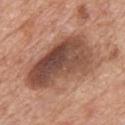Clinical impression:
Part of a total-body skin-imaging series; this lesion was reviewed on a skin check and was not flagged for biopsy.
Clinical summary:
A male patient aged 58–62. The total-body-photography lesion software estimated an eccentricity of roughly 0.85 and a shape-asymmetry score of about 0.3 (0 = symmetric). It also reported an average lesion color of about L≈49 a*≈21 b*≈28 (CIELAB), a lesion–skin lightness drop of about 14, and a normalized border contrast of about 10. The analysis additionally found a nevus-likeness score of about 0/100 and a lesion-detection confidence of about 100/100. A 15 mm close-up tile from a total-body photography series done for melanoma screening. This is a white-light tile. The lesion is on the chest. About 9.5 mm across.